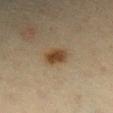follow-up — imaged on a skin check; not biopsied | lesion size — ~3 mm (longest diameter) | location — the right lower leg | patient — female, aged 63–67 | image source — ~15 mm crop, total-body skin-cancer survey.